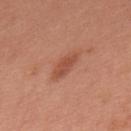{
  "biopsy_status": "not biopsied; imaged during a skin examination",
  "lighting": "white-light",
  "patient": {
    "sex": "male",
    "age_approx": 70
  },
  "image": {
    "source": "total-body photography crop",
    "field_of_view_mm": 15
  },
  "automated_metrics": {
    "vs_skin_darker_L": 9.0,
    "vs_skin_contrast_norm": 6.5,
    "nevus_likeness_0_100": 90,
    "lesion_detection_confidence_0_100": 100
  },
  "lesion_size": {
    "long_diameter_mm_approx": 4.0
  },
  "site": "upper back"
}